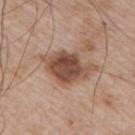workup — no biopsy performed (imaged during a skin exam); lesion diameter — ~5.5 mm (longest diameter); subject — male, about 65 years old; body site — the arm; image source — total-body-photography crop, ~15 mm field of view.